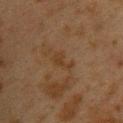{"biopsy_status": "not biopsied; imaged during a skin examination", "image": {"source": "total-body photography crop", "field_of_view_mm": 15}, "site": "back", "patient": {"sex": "female", "age_approx": 40}, "lesion_size": {"long_diameter_mm_approx": 3.0}}